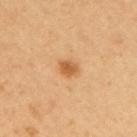Impression: Part of a total-body skin-imaging series; this lesion was reviewed on a skin check and was not flagged for biopsy. Image and clinical context: The recorded lesion diameter is about 2 mm. The lesion is located on the right upper arm. A female patient aged around 50. A 15 mm close-up extracted from a 3D total-body photography capture. Imaged with cross-polarized lighting.